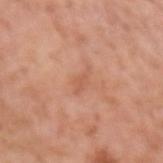Clinical impression:
The lesion was photographed on a routine skin check and not biopsied; there is no pathology result.
Background:
About 3 mm across. A lesion tile, about 15 mm wide, cut from a 3D total-body photograph. The patient is a male aged 73 to 77. Located on the arm. Captured under white-light illumination. An algorithmic analysis of the crop reported a footprint of about 3.5 mm², an outline eccentricity of about 0.85 (0 = round, 1 = elongated), and a shape-asymmetry score of about 0.3 (0 = symmetric). The software also gave a within-lesion color-variation index near 1/10 and peripheral color asymmetry of about 0.5.